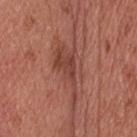No biopsy was performed on this lesion — it was imaged during a full skin examination and was not determined to be concerning.
The lesion is located on the head or neck.
An algorithmic analysis of the crop reported a footprint of about 13 mm². It also reported a lesion color around L≈44 a*≈25 b*≈27 in CIELAB, a lesion–skin lightness drop of about 9, and a lesion-to-skin contrast of about 7 (normalized; higher = more distinct). The software also gave border irregularity of about 9 on a 0–10 scale, internal color variation of about 4 on a 0–10 scale, and a peripheral color-asymmetry measure near 1.5. The software also gave a detector confidence of about 95 out of 100 that the crop contains a lesion.
About 8 mm across.
A 15 mm close-up extracted from a 3D total-body photography capture.
The patient is a male roughly 55 years of age.